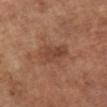Case summary:
• biopsy status · total-body-photography surveillance lesion; no biopsy
• lesion size · ~4 mm (longest diameter)
• imaging modality · ~15 mm tile from a whole-body skin photo
• anatomic site · the left lower leg
• patient · male, aged around 65